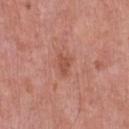{
  "biopsy_status": "not biopsied; imaged during a skin examination",
  "lighting": "white-light",
  "lesion_size": {
    "long_diameter_mm_approx": 3.0
  },
  "patient": {
    "sex": "male",
    "age_approx": 50
  },
  "site": "chest",
  "image": {
    "source": "total-body photography crop",
    "field_of_view_mm": 15
  }
}